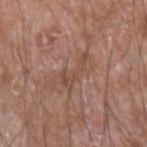notes: imaged on a skin check; not biopsied | size: about 4.5 mm | imaging modality: ~15 mm tile from a whole-body skin photo | body site: the arm | patient: male, aged 58 to 62.